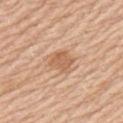Q: Was this lesion biopsied?
A: catalogued during a skin exam; not biopsied
Q: How was this image acquired?
A: total-body-photography crop, ~15 mm field of view
Q: Who is the patient?
A: male, about 60 years old
Q: Where on the body is the lesion?
A: the upper back
Q: What did automated image analysis measure?
A: a border-irregularity index near 2.5/10, a within-lesion color-variation index near 1.5/10, and a peripheral color-asymmetry measure near 0.5
Q: How was the tile lit?
A: white-light
Q: Lesion size?
A: about 2.5 mm